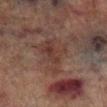follow-up=imaged on a skin check; not biopsied
lesion diameter=≈5.5 mm
subject=male, in their mid-70s
lighting=cross-polarized
acquisition=15 mm crop, total-body photography
anatomic site=the right lower leg